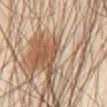Q: Illumination type?
A: cross-polarized
Q: Who is the patient?
A: male, about 45 years old
Q: Lesion size?
A: about 1 mm
Q: What is the imaging modality?
A: 15 mm crop, total-body photography
Q: Where on the body is the lesion?
A: the abdomen